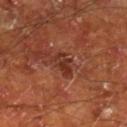Impression: Part of a total-body skin-imaging series; this lesion was reviewed on a skin check and was not flagged for biopsy. Clinical summary: The total-body-photography lesion software estimated lesion-presence confidence of about 100/100. A male patient, about 65 years old. A close-up tile cropped from a whole-body skin photograph, about 15 mm across. From the right lower leg. Approximately 3 mm at its widest. The tile uses cross-polarized illumination.